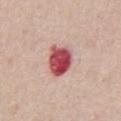| key | value |
|---|---|
| follow-up | imaged on a skin check; not biopsied |
| image | total-body-photography crop, ~15 mm field of view |
| subject | male, aged approximately 60 |
| lesion diameter | ≈4 mm |
| body site | the abdomen |